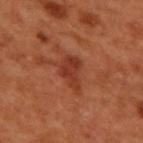Q: Was a biopsy performed?
A: imaged on a skin check; not biopsied
Q: Who is the patient?
A: male, aged around 50
Q: What did automated image analysis measure?
A: a lesion area of about 7.5 mm², a shape eccentricity near 0.8, and a shape-asymmetry score of about 0.4 (0 = symmetric); an average lesion color of about L≈38 a*≈30 b*≈33 (CIELAB), a lesion–skin lightness drop of about 9, and a lesion-to-skin contrast of about 7 (normalized; higher = more distinct); a border-irregularity rating of about 4.5/10 and a color-variation rating of about 2.5/10; a nevus-likeness score of about 5/100
Q: What kind of image is this?
A: ~15 mm tile from a whole-body skin photo
Q: What is the anatomic site?
A: the upper back
Q: Illumination type?
A: cross-polarized illumination